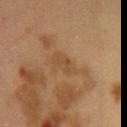Notes:
– workup — total-body-photography surveillance lesion; no biopsy
– anatomic site — the chest
– subject — female, in their 40s
– imaging modality — ~15 mm crop, total-body skin-cancer survey